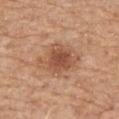follow-up: total-body-photography surveillance lesion; no biopsy
patient: female, approximately 70 years of age
location: the upper back
acquisition: 15 mm crop, total-body photography